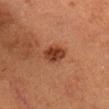Q: Was this lesion biopsied?
A: total-body-photography surveillance lesion; no biopsy
Q: What lighting was used for the tile?
A: cross-polarized
Q: What did automated image analysis measure?
A: a footprint of about 7 mm², an eccentricity of roughly 0.6, and a symmetry-axis asymmetry near 0.2; a border-irregularity rating of about 2/10 and internal color variation of about 3 on a 0–10 scale; a classifier nevus-likeness of about 95/100 and lesion-presence confidence of about 100/100
Q: What is the anatomic site?
A: the head or neck
Q: How large is the lesion?
A: about 3 mm
Q: Patient demographics?
A: female, aged around 70
Q: How was this image acquired?
A: ~15 mm tile from a whole-body skin photo